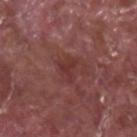Recorded during total-body skin imaging; not selected for excision or biopsy. A close-up tile cropped from a whole-body skin photograph, about 15 mm across. Longest diameter approximately 3 mm. Imaged with white-light lighting. Located on the left forearm. A male subject roughly 40 years of age.